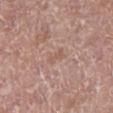Clinical impression:
Captured during whole-body skin photography for melanoma surveillance; the lesion was not biopsied.
Acquisition and patient details:
A female patient aged approximately 75. Imaged with white-light lighting. A lesion tile, about 15 mm wide, cut from a 3D total-body photograph. The lesion is on the leg.